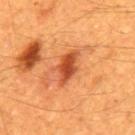<tbp_lesion>
  <biopsy_status>not biopsied; imaged during a skin examination</biopsy_status>
  <automated_metrics>
    <area_mm2_approx>7.5</area_mm2_approx>
    <eccentricity>0.85</eccentricity>
    <color_variation_0_10>3.0</color_variation_0_10>
    <peripheral_color_asymmetry>1.0</peripheral_color_asymmetry>
    <nevus_likeness_0_100>95</nevus_likeness_0_100>
    <lesion_detection_confidence_0_100>100</lesion_detection_confidence_0_100>
  </automated_metrics>
  <lighting>cross-polarized</lighting>
  <patient>
    <sex>male</sex>
    <age_approx>60</age_approx>
  </patient>
  <site>back</site>
  <image>
    <source>total-body photography crop</source>
    <field_of_view_mm>15</field_of_view_mm>
  </image>
</tbp_lesion>